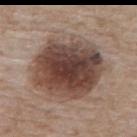Recorded during total-body skin imaging; not selected for excision or biopsy. On the upper back. Imaged with white-light lighting. Cropped from a total-body skin-imaging series; the visible field is about 15 mm. The lesion's longest dimension is about 9 mm. The subject is a female in their mid-70s.